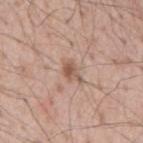No biopsy was performed on this lesion — it was imaged during a full skin examination and was not determined to be concerning.